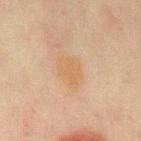Case summary:
* image: 15 mm crop, total-body photography
* tile lighting: cross-polarized illumination
* body site: the chest
* lesion size: ≈3.5 mm
* patient: female, aged around 50